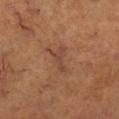No biopsy was performed on this lesion — it was imaged during a full skin examination and was not determined to be concerning. Longest diameter approximately 3.5 mm. A 15 mm close-up extracted from a 3D total-body photography capture. A female subject aged around 45. The lesion is located on the left leg.